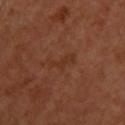No biopsy was performed on this lesion — it was imaged during a full skin examination and was not determined to be concerning. This image is a 15 mm lesion crop taken from a total-body photograph. Measured at roughly 3 mm in maximum diameter. Automated image analysis of the tile measured a mean CIELAB color near L≈33 a*≈23 b*≈31 and a normalized lesion–skin contrast near 5.5. And it measured a border-irregularity index near 6/10, a color-variation rating of about 0/10, and peripheral color asymmetry of about 0. Located on the upper back. Captured under cross-polarized illumination. The patient is a female aged 38 to 42.